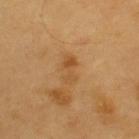Case summary:
- follow-up · no biopsy performed (imaged during a skin exam)
- illumination · cross-polarized
- image · ~15 mm tile from a whole-body skin photo
- patient · male, roughly 60 years of age
- size · about 3 mm
- automated metrics · a lesion color around L≈51 a*≈21 b*≈40 in CIELAB and about 7 CIELAB-L* units darker than the surrounding skin; a within-lesion color-variation index near 2.5/10 and peripheral color asymmetry of about 0.5
- body site · the upper back